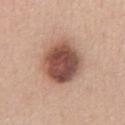Case summary:
• workup · imaged on a skin check; not biopsied
• location · the chest
• automated lesion analysis · two-axis asymmetry of about 0.1; a mean CIELAB color near L≈50 a*≈22 b*≈26, about 18 CIELAB-L* units darker than the surrounding skin, and a normalized border contrast of about 11.5
• subject · male, aged around 50
• size · about 5.5 mm
• tile lighting · white-light
• image · ~15 mm tile from a whole-body skin photo